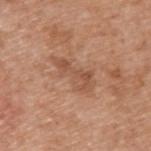{
  "patient": {
    "sex": "male",
    "age_approx": 65
  },
  "lesion_size": {
    "long_diameter_mm_approx": 5.5
  },
  "image": {
    "source": "total-body photography crop",
    "field_of_view_mm": 15
  },
  "site": "upper back",
  "automated_metrics": {
    "eccentricity": 0.9,
    "shape_asymmetry": 0.4,
    "cielab_L": 53,
    "cielab_a": 22,
    "cielab_b": 32,
    "vs_skin_darker_L": 8.0,
    "vs_skin_contrast_norm": 5.5
  }
}